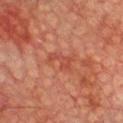The recorded lesion diameter is about 3.5 mm.
From the chest.
This image is a 15 mm lesion crop taken from a total-body photograph.
A male patient aged around 75.